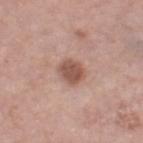The lesion was tiled from a total-body skin photograph and was not biopsied.
A roughly 15 mm field-of-view crop from a total-body skin photograph.
Located on the right thigh.
A female patient, aged 63 to 67.